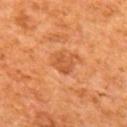No biopsy was performed on this lesion — it was imaged during a full skin examination and was not determined to be concerning.
The tile uses cross-polarized illumination.
The lesion is on the mid back.
Approximately 2.5 mm at its widest.
A roughly 15 mm field-of-view crop from a total-body skin photograph.
A male patient, roughly 65 years of age.
Automated tile analysis of the lesion measured an eccentricity of roughly 0.6 and two-axis asymmetry of about 0.2.